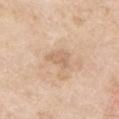Impression: The lesion was tiled from a total-body skin photograph and was not biopsied. Clinical summary: Cropped from a whole-body photographic skin survey; the tile spans about 15 mm. This is a white-light tile. About 3 mm across. From the left upper arm. The patient is a female about 75 years old.